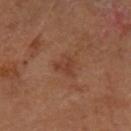Q: Is there a histopathology result?
A: imaged on a skin check; not biopsied
Q: Lesion location?
A: the left forearm
Q: What is the lesion's diameter?
A: ≈2.5 mm
Q: What kind of image is this?
A: 15 mm crop, total-body photography
Q: Who is the patient?
A: female, aged 58 to 62
Q: Automated lesion metrics?
A: a footprint of about 4 mm² and a shape eccentricity near 0.4